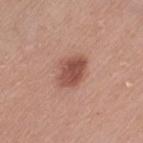Findings:
- notes: no biopsy performed (imaged during a skin exam)
- acquisition: 15 mm crop, total-body photography
- anatomic site: the leg
- TBP lesion metrics: a mean CIELAB color near L≈51 a*≈24 b*≈27 and a normalized border contrast of about 8.5
- lighting: white-light
- subject: female, roughly 55 years of age